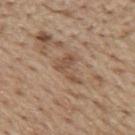workup — no biopsy performed (imaged during a skin exam) | image — total-body-photography crop, ~15 mm field of view | image-analysis metrics — a mean CIELAB color near L≈50 a*≈17 b*≈30, roughly 8 lightness units darker than nearby skin, and a normalized lesion–skin contrast near 6.5; a nevus-likeness score of about 0/100 and lesion-presence confidence of about 90/100 | subject — male, aged 68 to 72 | lesion diameter — ≈3.5 mm | site — the mid back.